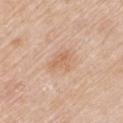Clinical impression:
Imaged during a routine full-body skin examination; the lesion was not biopsied and no histopathology is available.
Background:
The subject is a male roughly 80 years of age. The lesion is on the right upper arm. A 15 mm close-up tile from a total-body photography series done for melanoma screening.